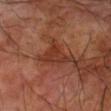Part of a total-body skin-imaging series; this lesion was reviewed on a skin check and was not flagged for biopsy. The recorded lesion diameter is about 3.5 mm. The patient is a male about 70 years old. On the right forearm. Captured under cross-polarized illumination. A roughly 15 mm field-of-view crop from a total-body skin photograph.